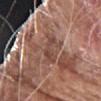{
  "biopsy_status": "not biopsied; imaged during a skin examination",
  "patient": {
    "sex": "male",
    "age_approx": 75
  },
  "site": "right forearm",
  "lighting": "white-light",
  "automated_metrics": {
    "cielab_L": 45,
    "cielab_a": 21,
    "cielab_b": 25,
    "vs_skin_darker_L": 8.0,
    "vs_skin_contrast_norm": 6.5,
    "nevus_likeness_0_100": 0,
    "lesion_detection_confidence_0_100": 65
  },
  "image": {
    "source": "total-body photography crop",
    "field_of_view_mm": 15
  },
  "lesion_size": {
    "long_diameter_mm_approx": 3.0
  }
}